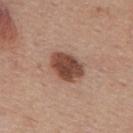Impression:
The lesion was photographed on a routine skin check and not biopsied; there is no pathology result.
Image and clinical context:
About 4.5 mm across. A 15 mm crop from a total-body photograph taken for skin-cancer surveillance. A male subject in their mid- to late 50s. Located on the mid back. Automated tile analysis of the lesion measured a detector confidence of about 100 out of 100 that the crop contains a lesion. Captured under white-light illumination.